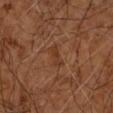Q: Was a biopsy performed?
A: no biopsy performed (imaged during a skin exam)
Q: Patient demographics?
A: aged 63 to 67
Q: Illumination type?
A: cross-polarized
Q: What is the anatomic site?
A: the right upper arm
Q: What kind of image is this?
A: ~15 mm crop, total-body skin-cancer survey